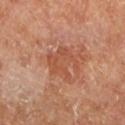Case summary:
- follow-up: no biopsy performed (imaged during a skin exam)
- size: about 4 mm
- illumination: cross-polarized
- subject: female
- location: the left lower leg
- image-analysis metrics: a mean CIELAB color near L≈53 a*≈27 b*≈35, roughly 7 lightness units darker than nearby skin, and a lesion-to-skin contrast of about 5.5 (normalized; higher = more distinct)
- image: total-body-photography crop, ~15 mm field of view